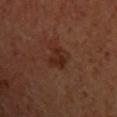<tbp_lesion>
  <biopsy_status>not biopsied; imaged during a skin examination</biopsy_status>
</tbp_lesion>